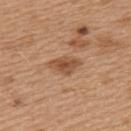follow-up = imaged on a skin check; not biopsied
tile lighting = white-light
lesion diameter = ~4 mm (longest diameter)
subject = female, aged 38–42
image source = ~15 mm tile from a whole-body skin photo
site = the left upper arm